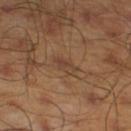Case summary:
• follow-up — no biopsy performed (imaged during a skin exam)
• subject — male, about 45 years old
• lighting — cross-polarized
• size — about 3 mm
• body site — the left lower leg
• acquisition — total-body-photography crop, ~15 mm field of view